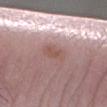{"biopsy_status": "not biopsied; imaged during a skin examination", "lighting": "white-light", "site": "arm", "lesion_size": {"long_diameter_mm_approx": 3.5}, "image": {"source": "total-body photography crop", "field_of_view_mm": 15}, "patient": {"sex": "male", "age_approx": 60}}